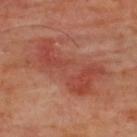The lesion was photographed on a routine skin check and not biopsied; there is no pathology result. The lesion is located on the upper back. The lesion's longest dimension is about 8.5 mm. The lesion-visualizer software estimated a border-irregularity rating of about 5.5/10, a color-variation rating of about 4/10, and peripheral color asymmetry of about 1. The tile uses cross-polarized illumination. A 15 mm crop from a total-body photograph taken for skin-cancer surveillance. A male patient about 65 years old.